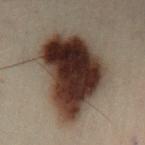image-analysis metrics: a lesion color around L≈25 a*≈13 b*≈18 in CIELAB, a lesion–skin lightness drop of about 20, and a normalized lesion–skin contrast near 18.5; a border-irregularity rating of about 3/10, a within-lesion color-variation index near 9/10, and radial color variation of about 3
acquisition: ~15 mm crop, total-body skin-cancer survey
lesion size: ≈9.5 mm
illumination: cross-polarized
anatomic site: the left lower leg
subject: male, in their 50s
histopathology: a dysplastic (Clark) nevus — a benign lesion